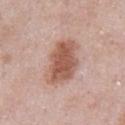This lesion was catalogued during total-body skin photography and was not selected for biopsy.
Captured under white-light illumination.
A male patient, aged approximately 55.
Cropped from a whole-body photographic skin survey; the tile spans about 15 mm.
The lesion is located on the front of the torso.
The lesion-visualizer software estimated an eccentricity of roughly 0.8 and two-axis asymmetry of about 0.25. And it measured a border-irregularity rating of about 2.5/10, internal color variation of about 4 on a 0–10 scale, and peripheral color asymmetry of about 1.